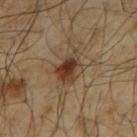Assessment:
Part of a total-body skin-imaging series; this lesion was reviewed on a skin check and was not flagged for biopsy.
Context:
Imaged with cross-polarized lighting. The subject is a male in their mid- to late 60s. Approximately 4.5 mm at its widest. Cropped from a total-body skin-imaging series; the visible field is about 15 mm. An algorithmic analysis of the crop reported a mean CIELAB color near L≈36 a*≈16 b*≈27 and a lesion-to-skin contrast of about 9.5 (normalized; higher = more distinct). The lesion is on the upper back.